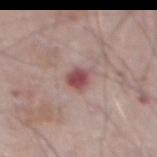Measured at roughly 2.5 mm in maximum diameter.
The tile uses white-light illumination.
A male patient, aged approximately 75.
Located on the abdomen.
A 15 mm close-up extracted from a 3D total-body photography capture.